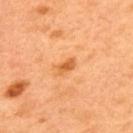Findings:
- size · ≈2.5 mm
- illumination · cross-polarized illumination
- anatomic site · the upper back
- subject · male, aged 48 to 52
- TBP lesion metrics · a mean CIELAB color near L≈62 a*≈29 b*≈48 and roughly 11 lightness units darker than nearby skin; border irregularity of about 2.5 on a 0–10 scale and radial color variation of about 0
- imaging modality · ~15 mm tile from a whole-body skin photo